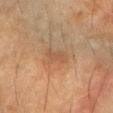{"biopsy_status": "not biopsied; imaged during a skin examination", "lighting": "cross-polarized", "site": "right forearm", "image": {"source": "total-body photography crop", "field_of_view_mm": 15}, "patient": {"sex": "female", "age_approx": 70}, "automated_metrics": {"vs_skin_darker_L": 6.0, "vs_skin_contrast_norm": 5.0}, "lesion_size": {"long_diameter_mm_approx": 3.0}}